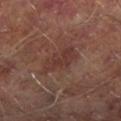<tbp_lesion>
  <biopsy_status>not biopsied; imaged during a skin examination</biopsy_status>
  <image>
    <source>total-body photography crop</source>
    <field_of_view_mm>15</field_of_view_mm>
  </image>
  <patient>
    <sex>male</sex>
    <age_approx>65</age_approx>
  </patient>
  <site>left lower leg</site>
</tbp_lesion>